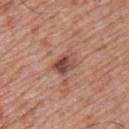| feature | finding |
|---|---|
| follow-up | catalogued during a skin exam; not biopsied |
| acquisition | total-body-photography crop, ~15 mm field of view |
| diameter | about 3 mm |
| site | the upper back |
| automated lesion analysis | a classifier nevus-likeness of about 70/100 |
| subject | male, approximately 65 years of age |